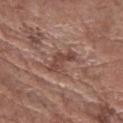workup: catalogued during a skin exam; not biopsied
patient: male, about 75 years old
image: 15 mm crop, total-body photography
location: the right forearm